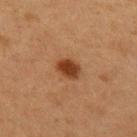– workup · catalogued during a skin exam; not biopsied
– site · the left upper arm
– patient · female, aged approximately 40
– acquisition · 15 mm crop, total-body photography
– size · ~3 mm (longest diameter)
– illumination · cross-polarized illumination